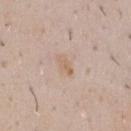– biopsy status — catalogued during a skin exam; not biopsied
– acquisition — 15 mm crop, total-body photography
– body site — the chest
– patient — male, aged 48 to 52